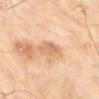Q: Who is the patient?
A: male, aged approximately 65
Q: How was this image acquired?
A: 15 mm crop, total-body photography
Q: What is the anatomic site?
A: the right lower leg
Q: Automated lesion metrics?
A: a footprint of about 5 mm² and a symmetry-axis asymmetry near 0.3; internal color variation of about 2 on a 0–10 scale and a peripheral color-asymmetry measure near 0.5; a classifier nevus-likeness of about 0/100 and lesion-presence confidence of about 100/100
Q: Lesion size?
A: ≈3.5 mm